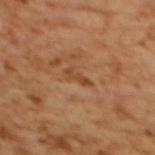biopsy_status: not biopsied; imaged during a skin examination
patient:
  sex: female
  age_approx: 55
lighting: cross-polarized
site: back
lesion_size:
  long_diameter_mm_approx: 3.0
image:
  source: total-body photography crop
  field_of_view_mm: 15
automated_metrics:
  area_mm2_approx: 2.5
  eccentricity: 0.9
  shape_asymmetry: 0.4
  border_irregularity_0_10: 5.5
  color_variation_0_10: 0.0
  peripheral_color_asymmetry: 0.0